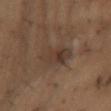Part of a total-body skin-imaging series; this lesion was reviewed on a skin check and was not flagged for biopsy. The lesion-visualizer software estimated an area of roughly 11 mm² and a shape-asymmetry score of about 0.2 (0 = symmetric). And it measured a lesion color around L≈30 a*≈12 b*≈20 in CIELAB, roughly 6 lightness units darker than nearby skin, and a normalized lesion–skin contrast near 6. On the left upper arm. Approximately 5.5 mm at its widest. A female subject aged around 55. A 15 mm crop from a total-body photograph taken for skin-cancer surveillance.